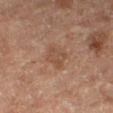Imaged with cross-polarized lighting. Cropped from a whole-body photographic skin survey; the tile spans about 15 mm. The patient is a female aged 53–57. The lesion is located on the right thigh. Approximately 3 mm at its widest.